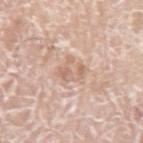<record>
  <biopsy_status>not biopsied; imaged during a skin examination</biopsy_status>
  <patient>
    <sex>male</sex>
    <age_approx>75</age_approx>
  </patient>
  <image>
    <source>total-body photography crop</source>
    <field_of_view_mm>15</field_of_view_mm>
  </image>
  <site>left upper arm</site>
</record>